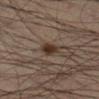Cropped from a total-body skin-imaging series; the visible field is about 15 mm.
This is a cross-polarized tile.
Located on the right lower leg.
The subject is a male aged approximately 35.